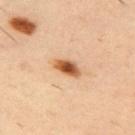Notes:
* biopsy status — no biopsy performed (imaged during a skin exam)
* body site — the upper back
* imaging modality — ~15 mm crop, total-body skin-cancer survey
* automated metrics — an area of roughly 5 mm², an eccentricity of roughly 0.85, and two-axis asymmetry of about 0.25; a mean CIELAB color near L≈56 a*≈24 b*≈39 and roughly 17 lightness units darker than nearby skin
* patient — male, roughly 40 years of age